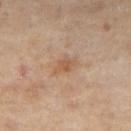{"patient": {"sex": "female", "age_approx": 50}, "site": "right thigh", "image": {"source": "total-body photography crop", "field_of_view_mm": 15}}